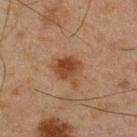Part of a total-body skin-imaging series; this lesion was reviewed on a skin check and was not flagged for biopsy. The lesion is located on the right lower leg. A close-up tile cropped from a whole-body skin photograph, about 15 mm across. The lesion-visualizer software estimated an eccentricity of roughly 0.25 and a shape-asymmetry score of about 0.25 (0 = symmetric). The software also gave a lesion color around L≈35 a*≈20 b*≈28 in CIELAB, a lesion–skin lightness drop of about 10, and a normalized border contrast of about 9.5. And it measured a nevus-likeness score of about 95/100 and lesion-presence confidence of about 100/100. A male patient about 45 years old.